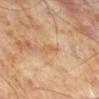Part of a total-body skin-imaging series; this lesion was reviewed on a skin check and was not flagged for biopsy.
From the right lower leg.
The recorded lesion diameter is about 3 mm.
A male subject aged approximately 70.
A 15 mm crop from a total-body photograph taken for skin-cancer surveillance.
This is a cross-polarized tile.